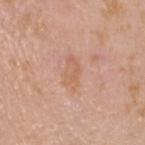Clinical impression: Part of a total-body skin-imaging series; this lesion was reviewed on a skin check and was not flagged for biopsy. Acquisition and patient details: The subject is a male aged approximately 25. The recorded lesion diameter is about 3.5 mm. This is a white-light tile. On the head or neck. A roughly 15 mm field-of-view crop from a total-body skin photograph.